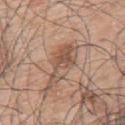Findings:
- workup: total-body-photography surveillance lesion; no biopsy
- tile lighting: white-light
- image: ~15 mm tile from a whole-body skin photo
- image-analysis metrics: a lesion area of about 9.5 mm²; a mean CIELAB color near L≈53 a*≈19 b*≈28, about 10 CIELAB-L* units darker than the surrounding skin, and a normalized border contrast of about 7; border irregularity of about 8 on a 0–10 scale, internal color variation of about 5 on a 0–10 scale, and radial color variation of about 1.5
- location: the upper back
- subject: male, aged 68 to 72